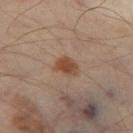Assessment:
This lesion was catalogued during total-body skin photography and was not selected for biopsy.
Image and clinical context:
Captured under cross-polarized illumination. On the left thigh. The subject is roughly 55 years of age. A 15 mm close-up extracted from a 3D total-body photography capture. Measured at roughly 3 mm in maximum diameter.